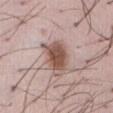site: abdomen
patient:
  sex: male
  age_approx: 50
image:
  source: total-body photography crop
  field_of_view_mm: 15